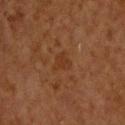follow-up=imaged on a skin check; not biopsied
image=~15 mm tile from a whole-body skin photo
subject=male, aged 63 to 67
anatomic site=the upper back